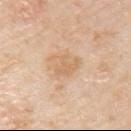Notes:
- follow-up · no biopsy performed (imaged during a skin exam)
- patient · male, approximately 75 years of age
- site · the arm
- imaging modality · ~15 mm crop, total-body skin-cancer survey
- illumination · white-light illumination